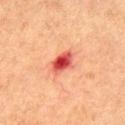The lesion was photographed on a routine skin check and not biopsied; there is no pathology result.
This is a cross-polarized tile.
Cropped from a whole-body photographic skin survey; the tile spans about 15 mm.
The total-body-photography lesion software estimated a lesion area of about 6 mm², an eccentricity of roughly 0.75, and two-axis asymmetry of about 0.2. The software also gave a lesion color around L≈48 a*≈37 b*≈31 in CIELAB, a lesion–skin lightness drop of about 15, and a normalized lesion–skin contrast near 10.5. And it measured border irregularity of about 2 on a 0–10 scale, internal color variation of about 7.5 on a 0–10 scale, and radial color variation of about 2. And it measured an automated nevus-likeness rating near 0 out of 100 and lesion-presence confidence of about 100/100.
A male patient approximately 65 years of age.
The lesion is located on the chest.